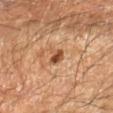follow-up — imaged on a skin check; not biopsied
image — total-body-photography crop, ~15 mm field of view
subject — male, aged 43–47
site — the left forearm
lesion size — about 2.5 mm